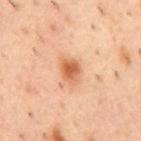Captured during whole-body skin photography for melanoma surveillance; the lesion was not biopsied. A 15 mm close-up tile from a total-body photography series done for melanoma screening. The tile uses cross-polarized illumination. The subject is a male aged approximately 55. The lesion's longest dimension is about 3 mm. The lesion is on the back.